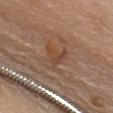Imaged during a routine full-body skin examination; the lesion was not biopsied and no histopathology is available. On the front of the torso. Imaged with white-light lighting. A region of skin cropped from a whole-body photographic capture, roughly 15 mm wide. Longest diameter approximately 3 mm. The patient is a male aged 58–62.